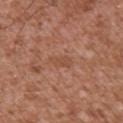workup: no biopsy performed (imaged during a skin exam)
lighting: white-light illumination
image source: ~15 mm crop, total-body skin-cancer survey
site: the chest
TBP lesion metrics: an area of roughly 3.5 mm²; a lesion color around L≈49 a*≈23 b*≈31 in CIELAB and a lesion-to-skin contrast of about 4.5 (normalized; higher = more distinct); a border-irregularity index near 3.5/10 and a within-lesion color-variation index near 0.5/10; lesion-presence confidence of about 95/100
diameter: ~3 mm (longest diameter)
subject: male, about 45 years old